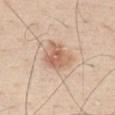Assessment: No biopsy was performed on this lesion — it was imaged during a full skin examination and was not determined to be concerning. Background: A 15 mm close-up tile from a total-body photography series done for melanoma screening. The lesion is on the chest. Approximately 4.5 mm at its widest. This is a white-light tile. Automated tile analysis of the lesion measured a footprint of about 13 mm² and a shape eccentricity near 0.45. The software also gave a mean CIELAB color near L≈64 a*≈19 b*≈30 and a lesion-to-skin contrast of about 6.5 (normalized; higher = more distinct). The analysis additionally found a color-variation rating of about 5/10 and radial color variation of about 1.5. A male patient, aged around 30.